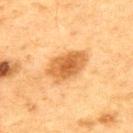  biopsy_status: not biopsied; imaged during a skin examination
  lesion_size:
    long_diameter_mm_approx: 5.0
  lighting: cross-polarized
  site: upper back
  image:
    source: total-body photography crop
    field_of_view_mm: 15
  patient:
    sex: male
    age_approx: 75